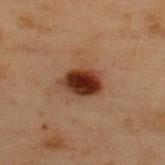The lesion was tiled from a total-body skin photograph and was not biopsied.
The lesion is on the back.
A close-up tile cropped from a whole-body skin photograph, about 15 mm across.
A male subject, approximately 55 years of age.